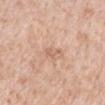Clinical impression: The lesion was tiled from a total-body skin photograph and was not biopsied. Clinical summary: Imaged with white-light lighting. On the mid back. An algorithmic analysis of the crop reported a detector confidence of about 100 out of 100 that the crop contains a lesion. A 15 mm crop from a total-body photograph taken for skin-cancer surveillance. Measured at roughly 2.5 mm in maximum diameter. A male patient aged approximately 50.